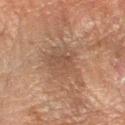The lesion is on the left forearm. A male subject, aged 63 to 67. This is a cross-polarized tile. Approximately 4.5 mm at its widest. A 15 mm crop from a total-body photograph taken for skin-cancer surveillance.